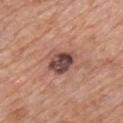notes: catalogued during a skin exam; not biopsied
tile lighting: white-light
patient: male, aged approximately 60
lesion diameter: about 3.5 mm
anatomic site: the chest
image source: ~15 mm tile from a whole-body skin photo